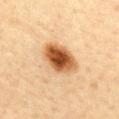Impression:
Recorded during total-body skin imaging; not selected for excision or biopsy.
Context:
On the mid back. Captured under cross-polarized illumination. The patient is a female in their mid-40s. The recorded lesion diameter is about 5 mm. A 15 mm close-up extracted from a 3D total-body photography capture. Automated tile analysis of the lesion measured a color-variation rating of about 7/10 and radial color variation of about 1.5. It also reported a classifier nevus-likeness of about 100/100 and a lesion-detection confidence of about 100/100.